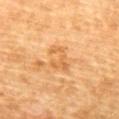* notes — imaged on a skin check; not biopsied
* TBP lesion metrics — an average lesion color of about L≈56 a*≈21 b*≈41 (CIELAB), roughly 7 lightness units darker than nearby skin, and a normalized border contrast of about 5.5; an automated nevus-likeness rating near 0 out of 100 and a detector confidence of about 100 out of 100 that the crop contains a lesion
* lesion diameter — ≈3.5 mm
* image source — ~15 mm crop, total-body skin-cancer survey
* subject — female, aged approximately 55
* location — the upper back